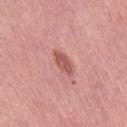patient: female, aged around 55; lesion size: about 4 mm; image: ~15 mm crop, total-body skin-cancer survey; site: the right thigh.